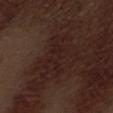Case summary:
– follow-up · imaged on a skin check; not biopsied
– image-analysis metrics · an area of roughly 10 mm², a shape eccentricity near 0.9, and a symmetry-axis asymmetry near 0.4; a detector confidence of about 60 out of 100 that the crop contains a lesion
– location · the abdomen
– illumination · white-light
– image source · ~15 mm crop, total-body skin-cancer survey
– patient · male, in their 70s